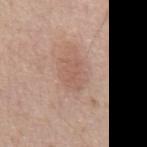workup = no biopsy performed (imaged during a skin exam) | subject = male, about 65 years old | lesion diameter = about 3.5 mm | acquisition = ~15 mm crop, total-body skin-cancer survey | tile lighting = white-light illumination | automated metrics = an area of roughly 5.5 mm² and an eccentricity of roughly 0.8; an average lesion color of about L≈57 a*≈20 b*≈27 (CIELAB), roughly 7 lightness units darker than nearby skin, and a normalized lesion–skin contrast near 4.5; a classifier nevus-likeness of about 5/100 and lesion-presence confidence of about 100/100 | location = the abdomen.